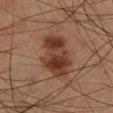workup: catalogued during a skin exam; not biopsied | size: ~6 mm (longest diameter) | location: the left lower leg | image: total-body-photography crop, ~15 mm field of view | subject: male, in their 60s | tile lighting: cross-polarized illumination.